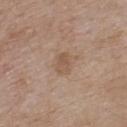Findings:
• workup — catalogued during a skin exam; not biopsied
• subject — male, aged around 75
• lesion diameter — ≈2.5 mm
• lighting — white-light illumination
• location — the back
• acquisition — ~15 mm tile from a whole-body skin photo